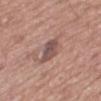Imaged during a routine full-body skin examination; the lesion was not biopsied and no histopathology is available.
A 15 mm close-up extracted from a 3D total-body photography capture.
Longest diameter approximately 4 mm.
Imaged with white-light lighting.
A male subject aged around 75.
Located on the mid back.